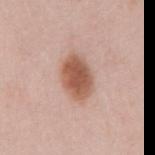Part of a total-body skin-imaging series; this lesion was reviewed on a skin check and was not flagged for biopsy.
Located on the mid back.
Measured at roughly 5 mm in maximum diameter.
This is a white-light tile.
Cropped from a whole-body photographic skin survey; the tile spans about 15 mm.
A female patient, about 40 years old.